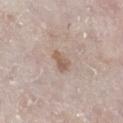Context:
A roughly 15 mm field-of-view crop from a total-body skin photograph. Located on the right lower leg. The total-body-photography lesion software estimated an area of roughly 4 mm², an outline eccentricity of about 0.75 (0 = round, 1 = elongated), and two-axis asymmetry of about 0.3. And it measured a classifier nevus-likeness of about 20/100 and lesion-presence confidence of about 100/100. A male patient aged 78 to 82. This is a white-light tile.